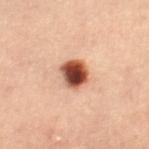follow-up=catalogued during a skin exam; not biopsied
acquisition=~15 mm crop, total-body skin-cancer survey
tile lighting=cross-polarized
patient=female, aged around 45
body site=the right thigh